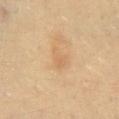Captured during whole-body skin photography for melanoma surveillance; the lesion was not biopsied.
Cropped from a total-body skin-imaging series; the visible field is about 15 mm.
On the lower back.
The recorded lesion diameter is about 2.5 mm.
The patient is a female aged 38–42.
The tile uses cross-polarized illumination.